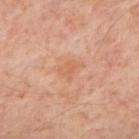| feature | finding |
|---|---|
| notes | no biopsy performed (imaged during a skin exam) |
| illumination | cross-polarized |
| image | total-body-photography crop, ~15 mm field of view |
| subject | aged 53 to 57 |
| anatomic site | the upper back |
| size | about 2.5 mm |